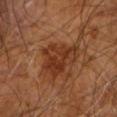Q: How large is the lesion?
A: about 5 mm
Q: How was this image acquired?
A: 15 mm crop, total-body photography
Q: Lesion location?
A: the left arm
Q: Illumination type?
A: cross-polarized illumination
Q: What are the patient's age and sex?
A: male, aged 58–62